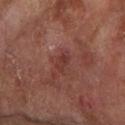Impression:
The lesion was photographed on a routine skin check and not biopsied; there is no pathology result.
Image and clinical context:
The patient is a male roughly 85 years of age or older. About 2.5 mm across. The tile uses cross-polarized illumination. The lesion is on the arm. A 15 mm crop from a total-body photograph taken for skin-cancer surveillance.